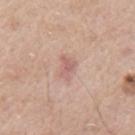The lesion was photographed on a routine skin check and not biopsied; there is no pathology result.